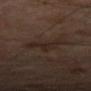Recorded during total-body skin imaging; not selected for excision or biopsy. A 15 mm close-up extracted from a 3D total-body photography capture. The total-body-photography lesion software estimated a footprint of about 11 mm², an outline eccentricity of about 0.85 (0 = round, 1 = elongated), and two-axis asymmetry of about 0.5. The software also gave a mean CIELAB color near L≈23 a*≈12 b*≈18 and about 4 CIELAB-L* units darker than the surrounding skin. And it measured a color-variation rating of about 3.5/10 and a peripheral color-asymmetry measure near 1. It also reported an automated nevus-likeness rating near 0 out of 100 and a lesion-detection confidence of about 95/100. The tile uses cross-polarized illumination. Located on the right thigh. The subject is a male aged 68 to 72. Approximately 5 mm at its widest.